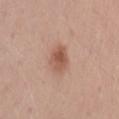  biopsy_status: not biopsied; imaged during a skin examination
  lesion_size:
    long_diameter_mm_approx: 3.5
  lighting: white-light
  image:
    source: total-body photography crop
    field_of_view_mm: 15
  patient:
    sex: male
    age_approx: 60
  site: left upper arm